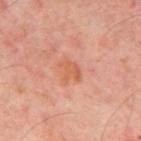Recorded during total-body skin imaging; not selected for excision or biopsy. An algorithmic analysis of the crop reported a mean CIELAB color near L≈59 a*≈28 b*≈36, roughly 7 lightness units darker than nearby skin, and a lesion-to-skin contrast of about 6 (normalized; higher = more distinct). The software also gave an automated nevus-likeness rating near 0 out of 100 and a detector confidence of about 100 out of 100 that the crop contains a lesion. The patient is aged around 55. The tile uses cross-polarized illumination. From the mid back. A roughly 15 mm field-of-view crop from a total-body skin photograph.